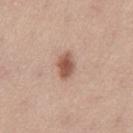| field | value |
|---|---|
| lighting | white-light |
| size | ≈3.5 mm |
| acquisition | total-body-photography crop, ~15 mm field of view |
| location | the leg |
| patient | female, aged 43–47 |